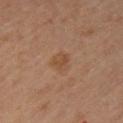This lesion was catalogued during total-body skin photography and was not selected for biopsy.
The total-body-photography lesion software estimated a lesion area of about 4.5 mm², an outline eccentricity of about 0.6 (0 = round, 1 = elongated), and a symmetry-axis asymmetry near 0.25. The software also gave a lesion color around L≈46 a*≈19 b*≈31 in CIELAB, a lesion–skin lightness drop of about 6, and a normalized lesion–skin contrast near 5.5.
A female patient aged around 45.
The lesion is on the left upper arm.
The tile uses cross-polarized illumination.
This image is a 15 mm lesion crop taken from a total-body photograph.
The lesion's longest dimension is about 2.5 mm.